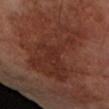  biopsy_status: not biopsied; imaged during a skin examination
  lesion_size:
    long_diameter_mm_approx: 10.0
  site: arm
  image:
    source: total-body photography crop
    field_of_view_mm: 15
  automated_metrics:
    border_irregularity_0_10: 9.5
    peripheral_color_asymmetry: 1.0
    lesion_detection_confidence_0_100: 90
  patient:
    sex: male
    age_approx: 70